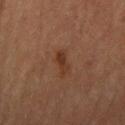The lesion was tiled from a total-body skin photograph and was not biopsied. A female patient in their 70s. A 15 mm close-up extracted from a 3D total-body photography capture. Automated image analysis of the tile measured a lesion color around L≈28 a*≈18 b*≈25 in CIELAB, roughly 6 lightness units darker than nearby skin, and a normalized lesion–skin contrast near 7. The lesion is on the right upper arm. Captured under cross-polarized illumination. About 3 mm across.